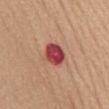Q: Is there a histopathology result?
A: total-body-photography surveillance lesion; no biopsy
Q: Patient demographics?
A: female, aged approximately 60
Q: Lesion size?
A: ≈3 mm
Q: Where on the body is the lesion?
A: the front of the torso
Q: Illumination type?
A: white-light illumination
Q: What kind of image is this?
A: 15 mm crop, total-body photography
Q: What did automated image analysis measure?
A: a lesion-to-skin contrast of about 12.5 (normalized; higher = more distinct); a within-lesion color-variation index near 6.5/10 and peripheral color asymmetry of about 2.5; an automated nevus-likeness rating near 0 out of 100 and lesion-presence confidence of about 100/100